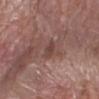anatomic site=the right forearm; subject=female, in their mid-60s; image=total-body-photography crop, ~15 mm field of view.